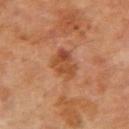The subject is a male aged around 70. Cropped from a total-body skin-imaging series; the visible field is about 15 mm. On the right upper arm. This is a cross-polarized tile. Automated image analysis of the tile measured a footprint of about 8 mm², an eccentricity of roughly 0.7, and a symmetry-axis asymmetry near 0.25. The software also gave internal color variation of about 5 on a 0–10 scale and radial color variation of about 1.5.